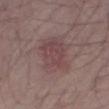Findings:
- biopsy status — total-body-photography surveillance lesion; no biopsy
- subject — male, aged 63 to 67
- size — about 5 mm
- image source — total-body-photography crop, ~15 mm field of view
- body site — the right thigh
- tile lighting — white-light illumination